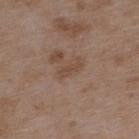The subject is a male about 50 years old.
A roughly 15 mm field-of-view crop from a total-body skin photograph.
On the upper back.